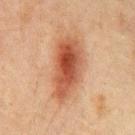Q: Is there a histopathology result?
A: imaged on a skin check; not biopsied